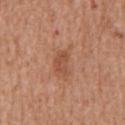Assessment:
Recorded during total-body skin imaging; not selected for excision or biopsy.
Context:
Cropped from a whole-body photographic skin survey; the tile spans about 15 mm. The lesion's longest dimension is about 3 mm. Imaged with white-light lighting. A male patient about 65 years old. From the chest.